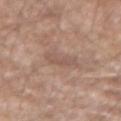{
  "biopsy_status": "not biopsied; imaged during a skin examination",
  "image": {
    "source": "total-body photography crop",
    "field_of_view_mm": 15
  },
  "site": "left forearm",
  "patient": {
    "sex": "male",
    "age_approx": 60
  },
  "lighting": "white-light",
  "lesion_size": {
    "long_diameter_mm_approx": 3.0
  }
}